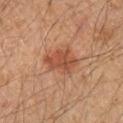Clinical impression:
Part of a total-body skin-imaging series; this lesion was reviewed on a skin check and was not flagged for biopsy.
Context:
About 4 mm across. A 15 mm crop from a total-body photograph taken for skin-cancer surveillance. A male subject aged 48 to 52. From the left forearm.